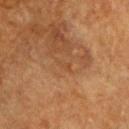The tile uses cross-polarized illumination. The lesion is located on the head or neck. Cropped from a whole-body photographic skin survey; the tile spans about 15 mm. The recorded lesion diameter is about 2 mm. A male subject, in their mid-80s or older.